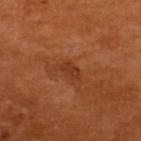<record>
  <patient>
    <sex>female</sex>
    <age_approx>55</age_approx>
  </patient>
  <image>
    <source>total-body photography crop</source>
    <field_of_view_mm>15</field_of_view_mm>
  </image>
  <lesion_size>
    <long_diameter_mm_approx>2.5</long_diameter_mm_approx>
  </lesion_size>
  <site>upper back</site>
  <lighting>cross-polarized</lighting>
</record>